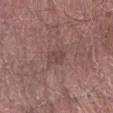<case>
<image>
  <source>total-body photography crop</source>
  <field_of_view_mm>15</field_of_view_mm>
</image>
<site>arm</site>
<lesion_size>
  <long_diameter_mm_approx>2.5</long_diameter_mm_approx>
</lesion_size>
<patient>
  <sex>male</sex>
  <age_approx>70</age_approx>
</patient>
<lighting>white-light</lighting>
</case>